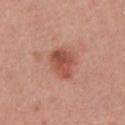This lesion was catalogued during total-body skin photography and was not selected for biopsy. A male subject aged 58–62. The tile uses white-light illumination. A 15 mm close-up tile from a total-body photography series done for melanoma screening. From the chest. About 4 mm across. Automated tile analysis of the lesion measured an area of roughly 8.5 mm², an outline eccentricity of about 0.65 (0 = round, 1 = elongated), and a shape-asymmetry score of about 0.25 (0 = symmetric). And it measured roughly 11 lightness units darker than nearby skin and a normalized lesion–skin contrast near 8. The analysis additionally found peripheral color asymmetry of about 1.5. The analysis additionally found a nevus-likeness score of about 90/100 and lesion-presence confidence of about 100/100.